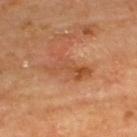Assessment:
The lesion was photographed on a routine skin check and not biopsied; there is no pathology result.
Image and clinical context:
The tile uses cross-polarized illumination. A 15 mm close-up tile from a total-body photography series done for melanoma screening. A male subject about 65 years old. Longest diameter approximately 5.5 mm. From the upper back. An algorithmic analysis of the crop reported a border-irregularity index near 7.5/10 and peripheral color asymmetry of about 1. And it measured an automated nevus-likeness rating near 0 out of 100 and a lesion-detection confidence of about 100/100.